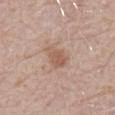{
  "biopsy_status": "not biopsied; imaged during a skin examination",
  "site": "abdomen",
  "image": {
    "source": "total-body photography crop",
    "field_of_view_mm": 15
  },
  "patient": {
    "sex": "male",
    "age_approx": 80
  }
}